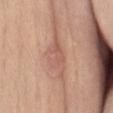{
  "biopsy_status": "not biopsied; imaged during a skin examination",
  "automated_metrics": {
    "area_mm2_approx": 9.0,
    "eccentricity": 0.9,
    "border_irregularity_0_10": 5.5,
    "color_variation_0_10": 3.0,
    "peripheral_color_asymmetry": 1.0,
    "nevus_likeness_0_100": 0,
    "lesion_detection_confidence_0_100": 100
  },
  "lesion_size": {
    "long_diameter_mm_approx": 6.0
  },
  "image": {
    "source": "total-body photography crop",
    "field_of_view_mm": 15
  },
  "site": "abdomen",
  "patient": {
    "sex": "female",
    "age_approx": 40
  }
}